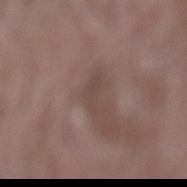Part of a total-body skin-imaging series; this lesion was reviewed on a skin check and was not flagged for biopsy. Cropped from a total-body skin-imaging series; the visible field is about 15 mm. Captured under white-light illumination. The patient is a male aged approximately 40. Approximately 3.5 mm at its widest. On the lower back.